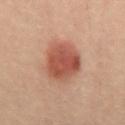The lesion's longest dimension is about 4.5 mm. A male patient, aged approximately 40. The tile uses cross-polarized illumination. Automated tile analysis of the lesion measured a lesion area of about 16 mm² and an outline eccentricity of about 0.4 (0 = round, 1 = elongated). It also reported roughly 10 lightness units darker than nearby skin and a normalized border contrast of about 8.5. The analysis additionally found border irregularity of about 1 on a 0–10 scale, a within-lesion color-variation index near 3.5/10, and a peripheral color-asymmetry measure near 1. A close-up tile cropped from a whole-body skin photograph, about 15 mm across. The lesion is located on the abdomen.